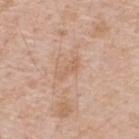Longest diameter approximately 3 mm. An algorithmic analysis of the crop reported an area of roughly 3 mm². The analysis additionally found a mean CIELAB color near L≈62 a*≈19 b*≈32, a lesion–skin lightness drop of about 6, and a lesion-to-skin contrast of about 5 (normalized; higher = more distinct). A 15 mm close-up tile from a total-body photography series done for melanoma screening. Located on the upper back. A male subject, about 50 years old.